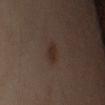workup: imaged on a skin check; not biopsied
lesion size: about 2.5 mm
patient: male, about 50 years old
illumination: cross-polarized illumination
imaging modality: total-body-photography crop, ~15 mm field of view
location: the arm